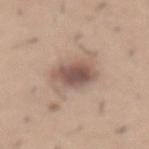workup=total-body-photography surveillance lesion; no biopsy | lesion diameter=~5.5 mm (longest diameter) | subject=male, aged 58–62 | lighting=white-light | body site=the abdomen | image source=~15 mm crop, total-body skin-cancer survey.